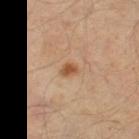Part of a total-body skin-imaging series; this lesion was reviewed on a skin check and was not flagged for biopsy. This image is a 15 mm lesion crop taken from a total-body photograph. Imaged with cross-polarized lighting. The recorded lesion diameter is about 2 mm. The patient is a male about 55 years old. The lesion is located on the leg.